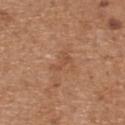<lesion>
  <biopsy_status>not biopsied; imaged during a skin examination</biopsy_status>
  <lesion_size>
    <long_diameter_mm_approx>2.5</long_diameter_mm_approx>
  </lesion_size>
  <site>upper back</site>
  <lighting>white-light</lighting>
  <patient>
    <sex>male</sex>
    <age_approx>70</age_approx>
  </patient>
  <image>
    <source>total-body photography crop</source>
    <field_of_view_mm>15</field_of_view_mm>
  </image>
</lesion>